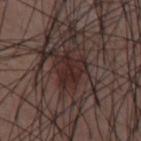notes — catalogued during a skin exam; not biopsied
body site — the abdomen
patient — male, aged approximately 50
image — ~15 mm tile from a whole-body skin photo